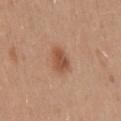The lesion was photographed on a routine skin check and not biopsied; there is no pathology result. The lesion's longest dimension is about 3.5 mm. This is a white-light tile. An algorithmic analysis of the crop reported an area of roughly 6.5 mm², an eccentricity of roughly 0.8, and two-axis asymmetry of about 0.2. It also reported a border-irregularity index near 2/10, a within-lesion color-variation index near 2.5/10, and radial color variation of about 1. The software also gave lesion-presence confidence of about 100/100. A roughly 15 mm field-of-view crop from a total-body skin photograph. A female patient, aged approximately 40. On the back.